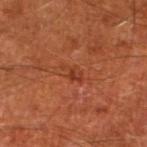follow-up: imaged on a skin check; not biopsied
illumination: cross-polarized illumination
image: ~15 mm tile from a whole-body skin photo
lesion size: ≈3 mm
location: the left lower leg
subject: male, aged approximately 65